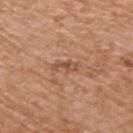Impression:
Recorded during total-body skin imaging; not selected for excision or biopsy.
Image and clinical context:
Cropped from a total-body skin-imaging series; the visible field is about 15 mm. A male patient, aged approximately 75. Located on the right upper arm.